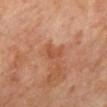Findings:
- biopsy status: total-body-photography surveillance lesion; no biopsy
- image source: total-body-photography crop, ~15 mm field of view
- subject: male, about 70 years old
- body site: the mid back